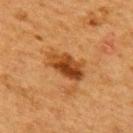No biopsy was performed on this lesion — it was imaged during a full skin examination and was not determined to be concerning. Imaged with cross-polarized lighting. Cropped from a whole-body photographic skin survey; the tile spans about 15 mm. The patient is a female roughly 50 years of age. Approximately 5 mm at its widest. From the upper back.